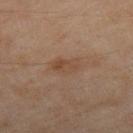<lesion>
<biopsy_status>not biopsied; imaged during a skin examination</biopsy_status>
<image>
  <source>total-body photography crop</source>
  <field_of_view_mm>15</field_of_view_mm>
</image>
<patient>
  <sex>female</sex>
  <age_approx>60</age_approx>
</patient>
<lighting>cross-polarized</lighting>
<site>leg</site>
<lesion_size>
  <long_diameter_mm_approx>3.5</long_diameter_mm_approx>
</lesion_size>
</lesion>